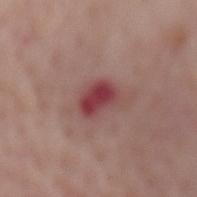Assessment: Part of a total-body skin-imaging series; this lesion was reviewed on a skin check and was not flagged for biopsy. Context: This is a white-light tile. An algorithmic analysis of the crop reported a lesion area of about 6 mm², a shape eccentricity near 0.75, and a shape-asymmetry score of about 0.25 (0 = symmetric). The analysis additionally found a lesion color around L≈41 a*≈30 b*≈20 in CIELAB, a lesion–skin lightness drop of about 12, and a lesion-to-skin contrast of about 10 (normalized; higher = more distinct). The software also gave an automated nevus-likeness rating near 0 out of 100 and a lesion-detection confidence of about 100/100. The lesion is located on the mid back. The subject is a female roughly 70 years of age. A region of skin cropped from a whole-body photographic capture, roughly 15 mm wide.